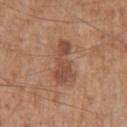Q: Was this lesion biopsied?
A: no biopsy performed (imaged during a skin exam)
Q: Illumination type?
A: white-light illumination
Q: What are the patient's age and sex?
A: male, aged 58–62
Q: Lesion location?
A: the right upper arm
Q: What is the imaging modality?
A: total-body-photography crop, ~15 mm field of view
Q: What did automated image analysis measure?
A: border irregularity of about 5.5 on a 0–10 scale, a color-variation rating of about 3/10, and a peripheral color-asymmetry measure near 1